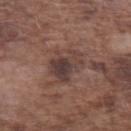Clinical impression: The lesion was tiled from a total-body skin photograph and was not biopsied. Background: The lesion's longest dimension is about 5 mm. A lesion tile, about 15 mm wide, cut from a 3D total-body photograph. The lesion is located on the arm. Captured under white-light illumination. The patient is a male approximately 75 years of age.